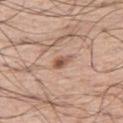Clinical impression:
Recorded during total-body skin imaging; not selected for excision or biopsy.
Context:
Automated tile analysis of the lesion measured a footprint of about 3 mm², an eccentricity of roughly 0.85, and two-axis asymmetry of about 0.3. The analysis additionally found a lesion color around L≈54 a*≈21 b*≈28 in CIELAB, a lesion–skin lightness drop of about 12, and a normalized lesion–skin contrast near 8.5. The software also gave border irregularity of about 2.5 on a 0–10 scale, a color-variation rating of about 2.5/10, and a peripheral color-asymmetry measure near 0.5. And it measured an automated nevus-likeness rating near 95 out of 100 and lesion-presence confidence of about 100/100. About 2.5 mm across. The subject is a male aged approximately 60. A 15 mm close-up extracted from a 3D total-body photography capture. Captured under white-light illumination. The lesion is located on the right thigh.